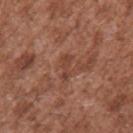– follow-up — imaged on a skin check; not biopsied
– acquisition — 15 mm crop, total-body photography
– location — the left upper arm
– lighting — white-light illumination
– subject — male, approximately 45 years of age